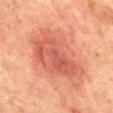The lesion was tiled from a total-body skin photograph and was not biopsied. A region of skin cropped from a whole-body photographic capture, roughly 15 mm wide. A male subject aged around 65. Approximately 8 mm at its widest. This is a cross-polarized tile. Located on the abdomen. An algorithmic analysis of the crop reported an average lesion color of about L≈47 a*≈26 b*≈27 (CIELAB) and a normalized lesion–skin contrast near 6.5. And it measured a nevus-likeness score of about 15/100.